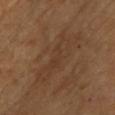An algorithmic analysis of the crop reported a shape eccentricity near 0.95 and two-axis asymmetry of about 0.4. And it measured a color-variation rating of about 2.5/10 and radial color variation of about 0.5. From the chest. Imaged with cross-polarized lighting. A 15 mm close-up tile from a total-body photography series done for melanoma screening. A male subject approximately 65 years of age.